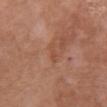The recorded lesion diameter is about 2.5 mm.
The tile uses white-light illumination.
The patient is a female about 70 years old.
Automated image analysis of the tile measured a mean CIELAB color near L≈49 a*≈24 b*≈31, about 6 CIELAB-L* units darker than the surrounding skin, and a normalized lesion–skin contrast near 4.5. And it measured an automated nevus-likeness rating near 0 out of 100.
On the chest.
Cropped from a total-body skin-imaging series; the visible field is about 15 mm.